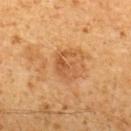Q: Was a biopsy performed?
A: total-body-photography surveillance lesion; no biopsy
Q: How was the tile lit?
A: cross-polarized
Q: How large is the lesion?
A: about 3 mm
Q: What is the imaging modality?
A: ~15 mm tile from a whole-body skin photo
Q: What is the anatomic site?
A: the upper back
Q: Who is the patient?
A: male, about 60 years old